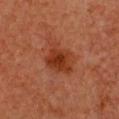<case>
<biopsy_status>not biopsied; imaged during a skin examination</biopsy_status>
<automated_metrics>
  <cielab_L>31</cielab_L>
  <cielab_a>25</cielab_a>
  <cielab_b>30</cielab_b>
  <vs_skin_contrast_norm>8.5</vs_skin_contrast_norm>
  <nevus_likeness_0_100>85</nevus_likeness_0_100>
</automated_metrics>
<image>
  <source>total-body photography crop</source>
  <field_of_view_mm>15</field_of_view_mm>
</image>
<lighting>cross-polarized</lighting>
<site>front of the torso</site>
<patient>
  <sex>female</sex>
  <age_approx>40</age_approx>
</patient>
</case>